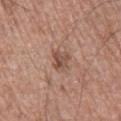| feature | finding |
|---|---|
| patient | male, roughly 60 years of age |
| diameter | ≈2.5 mm |
| tile lighting | white-light illumination |
| image source | ~15 mm crop, total-body skin-cancer survey |
| location | the right lower leg |